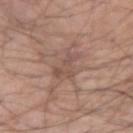follow-up: total-body-photography surveillance lesion; no biopsy
diameter: about 4.5 mm
anatomic site: the right forearm
automated lesion analysis: an outline eccentricity of about 0.85 (0 = round, 1 = elongated) and two-axis asymmetry of about 0.4
acquisition: total-body-photography crop, ~15 mm field of view
lighting: white-light illumination
patient: male, aged 48–52